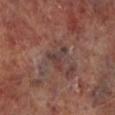Captured during whole-body skin photography for melanoma surveillance; the lesion was not biopsied. This is a cross-polarized tile. The recorded lesion diameter is about 3 mm. A 15 mm crop from a total-body photograph taken for skin-cancer surveillance. On the left lower leg. The subject is a male aged 63 to 67.